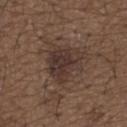Q: Was this lesion biopsied?
A: no biopsy performed (imaged during a skin exam)